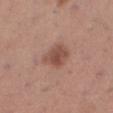Notes:
* biopsy status: imaged on a skin check; not biopsied
* body site: the left lower leg
* patient: female, in their 30s
* image: 15 mm crop, total-body photography
* TBP lesion metrics: a lesion area of about 8.5 mm², an eccentricity of roughly 0.6, and a shape-asymmetry score of about 0.15 (0 = symmetric); a lesion–skin lightness drop of about 10 and a normalized border contrast of about 7.5; a classifier nevus-likeness of about 90/100 and a lesion-detection confidence of about 100/100
* tile lighting: white-light
* lesion diameter: ≈3.5 mm